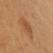No biopsy was performed on this lesion — it was imaged during a full skin examination and was not determined to be concerning.
A female patient, in their mid-40s.
The lesion's longest dimension is about 4.5 mm.
Captured under cross-polarized illumination.
On the front of the torso.
A 15 mm close-up tile from a total-body photography series done for melanoma screening.
Automated image analysis of the tile measured an average lesion color of about L≈51 a*≈22 b*≈38 (CIELAB) and a lesion-to-skin contrast of about 5 (normalized; higher = more distinct).